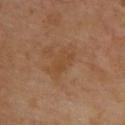Q: What are the patient's age and sex?
A: male, aged approximately 70
Q: Lesion location?
A: the upper back
Q: What lighting was used for the tile?
A: cross-polarized
Q: Automated lesion metrics?
A: a footprint of about 4.5 mm², an outline eccentricity of about 0.85 (0 = round, 1 = elongated), and a shape-asymmetry score of about 0.25 (0 = symmetric); border irregularity of about 3 on a 0–10 scale, a color-variation rating of about 2/10, and peripheral color asymmetry of about 0.5
Q: How was this image acquired?
A: ~15 mm crop, total-body skin-cancer survey